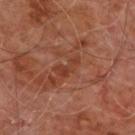Recorded during total-body skin imaging; not selected for excision or biopsy. Longest diameter approximately 3.5 mm. Cropped from a whole-body photographic skin survey; the tile spans about 15 mm. A male subject, approximately 60 years of age. The lesion-visualizer software estimated a lesion area of about 4.5 mm² and a symmetry-axis asymmetry near 0.5. It also reported a lesion–skin lightness drop of about 6 and a normalized lesion–skin contrast near 6. And it measured a nevus-likeness score of about 0/100 and lesion-presence confidence of about 100/100. The lesion is located on the chest.